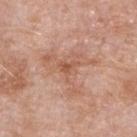Impression:
Recorded during total-body skin imaging; not selected for excision or biopsy.
Context:
A 15 mm close-up tile from a total-body photography series done for melanoma screening. On the arm. The lesion's longest dimension is about 6 mm. This is a white-light tile. The patient is a female in their 70s.